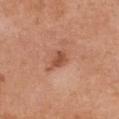biopsy_status: not biopsied; imaged during a skin examination
site: chest
lesion_size:
  long_diameter_mm_approx: 3.0
lighting: white-light
image:
  source: total-body photography crop
  field_of_view_mm: 15
patient:
  sex: female
  age_approx: 55